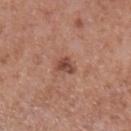<case>
<biopsy_status>not biopsied; imaged during a skin examination</biopsy_status>
<image>
  <source>total-body photography crop</source>
  <field_of_view_mm>15</field_of_view_mm>
</image>
<lesion_size>
  <long_diameter_mm_approx>2.5</long_diameter_mm_approx>
</lesion_size>
<automated_metrics>
  <area_mm2_approx>4.0</area_mm2_approx>
  <eccentricity>0.65</eccentricity>
  <shape_asymmetry>0.25</shape_asymmetry>
  <cielab_L>47</cielab_L>
  <cielab_a>24</cielab_a>
  <cielab_b>28</cielab_b>
  <border_irregularity_0_10>2.5</border_irregularity_0_10>
  <color_variation_0_10>3.5</color_variation_0_10>
  <peripheral_color_asymmetry>1.0</peripheral_color_asymmetry>
</automated_metrics>
<patient>
  <sex>male</sex>
  <age_approx>75</age_approx>
</patient>
<site>left lower leg</site>
<lighting>white-light</lighting>
</case>